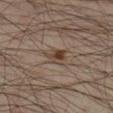Captured during whole-body skin photography for melanoma surveillance; the lesion was not biopsied. From the leg. A male patient aged around 35. Captured under cross-polarized illumination. The lesion's longest dimension is about 3 mm. A region of skin cropped from a whole-body photographic capture, roughly 15 mm wide.